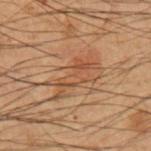workup: imaged on a skin check; not biopsied
location: the left forearm
diameter: ~5.5 mm (longest diameter)
subject: male, in their mid-50s
automated lesion analysis: a border-irregularity rating of about 7/10, a color-variation rating of about 3.5/10, and peripheral color asymmetry of about 1
acquisition: 15 mm crop, total-body photography
illumination: cross-polarized illumination